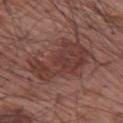Part of a total-body skin-imaging series; this lesion was reviewed on a skin check and was not flagged for biopsy. Cropped from a whole-body photographic skin survey; the tile spans about 15 mm. On the right upper arm. A male patient, aged approximately 65.